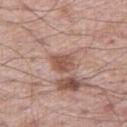Part of a total-body skin-imaging series; this lesion was reviewed on a skin check and was not flagged for biopsy. Cropped from a total-body skin-imaging series; the visible field is about 15 mm. The patient is a male about 60 years old. On the right thigh. The total-body-photography lesion software estimated a lesion color around L≈53 a*≈21 b*≈27 in CIELAB, roughly 11 lightness units darker than nearby skin, and a normalized lesion–skin contrast near 7.5. The analysis additionally found a nevus-likeness score of about 0/100 and lesion-presence confidence of about 100/100.